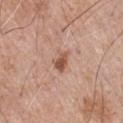| field | value |
|---|---|
| automated lesion analysis | an average lesion color of about L≈54 a*≈22 b*≈30 (CIELAB) and a lesion-to-skin contrast of about 8.5 (normalized; higher = more distinct); a border-irregularity rating of about 4/10, a color-variation rating of about 3/10, and peripheral color asymmetry of about 1; a classifier nevus-likeness of about 85/100 and lesion-presence confidence of about 100/100 |
| diameter | about 3 mm |
| illumination | white-light illumination |
| acquisition | 15 mm crop, total-body photography |
| body site | the chest |
| subject | male, aged approximately 65 |